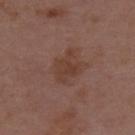Assessment: The lesion was tiled from a total-body skin photograph and was not biopsied. Clinical summary: On the upper back. The subject is a female aged around 45. A lesion tile, about 15 mm wide, cut from a 3D total-body photograph.